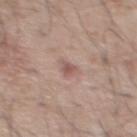Notes:
– workup: imaged on a skin check; not biopsied
– size: about 2 mm
– subject: male, in their mid- to late 50s
– anatomic site: the upper back
– imaging modality: total-body-photography crop, ~15 mm field of view
– tile lighting: white-light